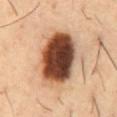This lesion was catalogued during total-body skin photography and was not selected for biopsy. A 15 mm close-up tile from a total-body photography series done for melanoma screening. The lesion is on the abdomen. A male subject, aged around 55.